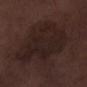{"biopsy_status": "not biopsied; imaged during a skin examination", "image": {"source": "total-body photography crop", "field_of_view_mm": 15}, "lighting": "white-light", "lesion_size": {"long_diameter_mm_approx": 8.5}, "automated_metrics": {"nevus_likeness_0_100": 0, "lesion_detection_confidence_0_100": 100}, "site": "left lower leg", "patient": {"sex": "male", "age_approx": 70}}